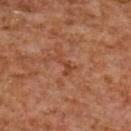Findings:
– workup — imaged on a skin check; not biopsied
– lighting — cross-polarized illumination
– patient — female, aged around 60
– acquisition — total-body-photography crop, ~15 mm field of view
– site — the upper back
– size — about 2.5 mm
– automated lesion analysis — an area of roughly 2.5 mm² and an outline eccentricity of about 0.9 (0 = round, 1 = elongated); a lesion color around L≈38 a*≈23 b*≈30 in CIELAB, a lesion–skin lightness drop of about 6, and a normalized lesion–skin contrast near 6; a within-lesion color-variation index near 0/10 and peripheral color asymmetry of about 0